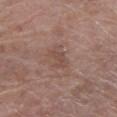workup = catalogued during a skin exam; not biopsied
acquisition = ~15 mm tile from a whole-body skin photo
body site = the right lower leg
patient = male, aged approximately 70